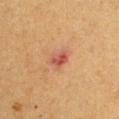Captured during whole-body skin photography for melanoma surveillance; the lesion was not biopsied. A 15 mm close-up tile from a total-body photography series done for melanoma screening. The patient is a male approximately 55 years of age. The lesion is on the left arm. Automated tile analysis of the lesion measured a lesion area of about 4 mm², a shape eccentricity near 0.7, and a shape-asymmetry score of about 0.3 (0 = symmetric). The analysis additionally found an average lesion color of about L≈48 a*≈26 b*≈28 (CIELAB), about 9 CIELAB-L* units darker than the surrounding skin, and a normalized border contrast of about 8.